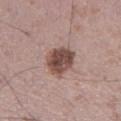<record>
<biopsy_status>not biopsied; imaged during a skin examination</biopsy_status>
<lesion_size>
  <long_diameter_mm_approx>4.5</long_diameter_mm_approx>
</lesion_size>
<lighting>white-light</lighting>
<patient>
  <sex>male</sex>
  <age_approx>50</age_approx>
</patient>
<site>leg</site>
<image>
  <source>total-body photography crop</source>
  <field_of_view_mm>15</field_of_view_mm>
</image>
<automated_metrics>
  <area_mm2_approx>11.0</area_mm2_approx>
  <eccentricity>0.65</eccentricity>
  <shape_asymmetry>0.2</shape_asymmetry>
  <border_irregularity_0_10>2.0</border_irregularity_0_10>
  <color_variation_0_10>5.5</color_variation_0_10>
  <peripheral_color_asymmetry>2.0</peripheral_color_asymmetry>
</automated_metrics>
</record>